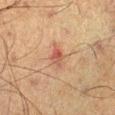Q: Was this lesion biopsied?
A: no biopsy performed (imaged during a skin exam)
Q: Lesion location?
A: the left lower leg
Q: What is the imaging modality?
A: ~15 mm tile from a whole-body skin photo
Q: Lesion size?
A: ≈3 mm
Q: Automated lesion metrics?
A: a classifier nevus-likeness of about 0/100 and a lesion-detection confidence of about 100/100
Q: Patient demographics?
A: male, aged 58–62
Q: What lighting was used for the tile?
A: cross-polarized illumination